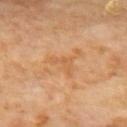  biopsy_status: not biopsied; imaged during a skin examination
  lesion_size:
    long_diameter_mm_approx: 3.0
  image:
    source: total-body photography crop
    field_of_view_mm: 15
  lighting: cross-polarized
  site: upper back